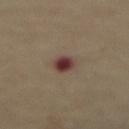Imaged during a routine full-body skin examination; the lesion was not biopsied and no histopathology is available.
A male subject aged 63–67.
Cropped from a whole-body photographic skin survey; the tile spans about 15 mm.
The tile uses cross-polarized illumination.
On the abdomen.